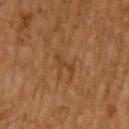follow-up: no biopsy performed (imaged during a skin exam) | imaging modality: ~15 mm crop, total-body skin-cancer survey | location: the left upper arm | lesion diameter: ~3 mm (longest diameter) | subject: male, aged approximately 60 | illumination: cross-polarized.